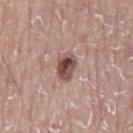Captured during whole-body skin photography for melanoma surveillance; the lesion was not biopsied. About 3.5 mm across. Captured under white-light illumination. A close-up tile cropped from a whole-body skin photograph, about 15 mm across. The patient is a male roughly 75 years of age. On the back.